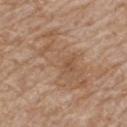biopsy status = no biopsy performed (imaged during a skin exam) | patient = male, aged around 80 | automated metrics = a footprint of about 20 mm² and an eccentricity of roughly 0.9; a border-irregularity rating of about 9/10 and radial color variation of about 1.5 | image source = ~15 mm crop, total-body skin-cancer survey | illumination = white-light illumination | site = the upper back | diameter = ~8.5 mm (longest diameter).